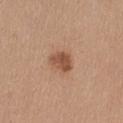No biopsy was performed on this lesion — it was imaged during a full skin examination and was not determined to be concerning.
Approximately 3 mm at its widest.
The lesion is located on the left thigh.
A female patient, aged around 40.
A region of skin cropped from a whole-body photographic capture, roughly 15 mm wide.
Imaged with white-light lighting.
Automated tile analysis of the lesion measured an eccentricity of roughly 0.65 and a symmetry-axis asymmetry near 0.35. And it measured a normalized border contrast of about 8.